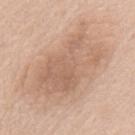Case summary:
* follow-up: total-body-photography surveillance lesion; no biopsy
* patient: female, approximately 75 years of age
* lesion diameter: ≈10.5 mm
* automated metrics: about 9 CIELAB-L* units darker than the surrounding skin and a normalized lesion–skin contrast near 6; border irregularity of about 4.5 on a 0–10 scale, a color-variation rating of about 4/10, and radial color variation of about 1.5
* imaging modality: ~15 mm crop, total-body skin-cancer survey
* anatomic site: the back